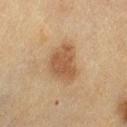Q: Is there a histopathology result?
A: total-body-photography surveillance lesion; no biopsy
Q: What lighting was used for the tile?
A: cross-polarized
Q: Who is the patient?
A: female, about 55 years old
Q: Lesion size?
A: ~4.5 mm (longest diameter)
Q: Automated lesion metrics?
A: a shape eccentricity near 0.65 and a shape-asymmetry score of about 0.3 (0 = symmetric); a border-irregularity rating of about 3/10, a color-variation rating of about 2.5/10, and radial color variation of about 1; a classifier nevus-likeness of about 85/100 and a detector confidence of about 100 out of 100 that the crop contains a lesion
Q: What is the anatomic site?
A: the left thigh
Q: What kind of image is this?
A: total-body-photography crop, ~15 mm field of view